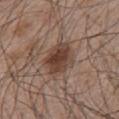Case summary:
– follow-up: no biopsy performed (imaged during a skin exam)
– illumination: white-light illumination
– TBP lesion metrics: an automated nevus-likeness rating near 90 out of 100
– patient: male, aged 63 to 67
– site: the chest
– acquisition: total-body-photography crop, ~15 mm field of view
– diameter: about 4.5 mm